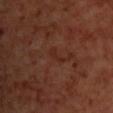{"biopsy_status": "not biopsied; imaged during a skin examination"}